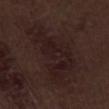{
  "biopsy_status": "not biopsied; imaged during a skin examination",
  "lighting": "white-light",
  "image": {
    "source": "total-body photography crop",
    "field_of_view_mm": 15
  },
  "patient": {
    "sex": "male",
    "age_approx": 70
  },
  "lesion_size": {
    "long_diameter_mm_approx": 9.5
  },
  "site": "left thigh"
}